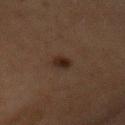Assessment:
The lesion was photographed on a routine skin check and not biopsied; there is no pathology result.
Background:
This is a cross-polarized tile. A 15 mm crop from a total-body photograph taken for skin-cancer surveillance. From the mid back. A female subject, roughly 60 years of age. The lesion-visualizer software estimated an automated nevus-likeness rating near 95 out of 100 and a detector confidence of about 100 out of 100 that the crop contains a lesion.